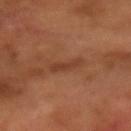  biopsy_status: not biopsied; imaged during a skin examination
  patient:
    sex: male
    age_approx: 65
  automated_metrics:
    border_irregularity_0_10: 3.5
    nevus_likeness_0_100: 0
    lesion_detection_confidence_0_100: 100
  image:
    source: total-body photography crop
    field_of_view_mm: 15